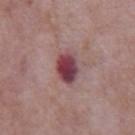– follow-up — no biopsy performed (imaged during a skin exam)
– image source — ~15 mm crop, total-body skin-cancer survey
– lesion size — about 4 mm
– anatomic site — the front of the torso
– lighting — white-light
– patient — male, aged around 65